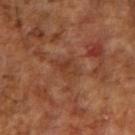No biopsy was performed on this lesion — it was imaged during a full skin examination and was not determined to be concerning. A male patient, approximately 65 years of age. The lesion is located on the right upper arm. Captured under cross-polarized illumination. A lesion tile, about 15 mm wide, cut from a 3D total-body photograph.